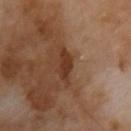patient: male, about 55 years old
TBP lesion metrics: a lesion color around L≈32 a*≈20 b*≈28 in CIELAB, about 9 CIELAB-L* units darker than the surrounding skin, and a lesion-to-skin contrast of about 8 (normalized; higher = more distinct)
tile lighting: cross-polarized
acquisition: ~15 mm crop, total-body skin-cancer survey
lesion size: ≈3.5 mm
body site: the chest